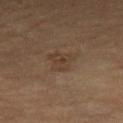workup: catalogued during a skin exam; not biopsied | illumination: cross-polarized | site: the left thigh | TBP lesion metrics: a mean CIELAB color near L≈36 a*≈14 b*≈25, roughly 5 lightness units darker than nearby skin, and a lesion-to-skin contrast of about 5 (normalized; higher = more distinct) | subject: female, about 70 years old | image: ~15 mm tile from a whole-body skin photo.